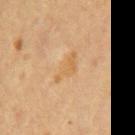Imaged during a routine full-body skin examination; the lesion was not biopsied and no histopathology is available. Located on the mid back. The lesion-visualizer software estimated an outline eccentricity of about 0.9 (0 = round, 1 = elongated) and a symmetry-axis asymmetry near 0.45. And it measured a border-irregularity index near 5.5/10, a within-lesion color-variation index near 1.5/10, and a peripheral color-asymmetry measure near 0.5. It also reported an automated nevus-likeness rating near 0 out of 100. A male patient, in their mid- to late 60s. Imaged with cross-polarized lighting. Measured at roughly 4 mm in maximum diameter. A close-up tile cropped from a whole-body skin photograph, about 15 mm across.